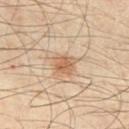  site: chest
  lesion_size:
    long_diameter_mm_approx: 2.5
  image:
    source: total-body photography crop
    field_of_view_mm: 15
  patient:
    sex: male
    age_approx: 55